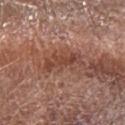Assessment:
Part of a total-body skin-imaging series; this lesion was reviewed on a skin check and was not flagged for biopsy.
Acquisition and patient details:
On the left forearm. A male patient in their 70s. A 15 mm crop from a total-body photograph taken for skin-cancer surveillance. The recorded lesion diameter is about 5 mm.